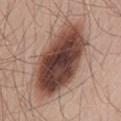Acquisition and patient details:
From the back. A male subject aged around 50. A 15 mm crop from a total-body photograph taken for skin-cancer surveillance. The total-body-photography lesion software estimated an eccentricity of roughly 0.85 and a symmetry-axis asymmetry near 0.15.